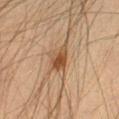• imaging modality — 15 mm crop, total-body photography
• automated metrics — a footprint of about 5.5 mm², a shape eccentricity near 0.8, and a shape-asymmetry score of about 0.35 (0 = symmetric); a border-irregularity index near 3.5/10 and peripheral color asymmetry of about 1; an automated nevus-likeness rating near 95 out of 100 and a lesion-detection confidence of about 100/100
• lighting — cross-polarized
• subject — male, aged approximately 35
• diameter — about 3.5 mm
• location — the right thigh